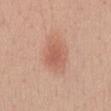Assessment: No biopsy was performed on this lesion — it was imaged during a full skin examination and was not determined to be concerning. Background: The lesion is located on the front of the torso. The total-body-photography lesion software estimated a border-irregularity index near 2.5/10, internal color variation of about 2 on a 0–10 scale, and radial color variation of about 0.5. Cropped from a total-body skin-imaging series; the visible field is about 15 mm. About 3 mm across. Captured under white-light illumination. A male patient, aged 28–32.